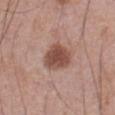biopsy status = no biopsy performed (imaged during a skin exam)
image-analysis metrics = a lesion color around L≈49 a*≈22 b*≈25 in CIELAB and about 13 CIELAB-L* units darker than the surrounding skin; a border-irregularity index near 2/10, a color-variation rating of about 3/10, and radial color variation of about 1; an automated nevus-likeness rating near 95 out of 100 and a lesion-detection confidence of about 100/100
tile lighting = white-light
imaging modality = ~15 mm crop, total-body skin-cancer survey
patient = male, roughly 70 years of age
anatomic site = the abdomen
size = ~4 mm (longest diameter)